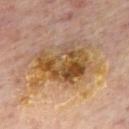Q: Was a biopsy performed?
A: imaged on a skin check; not biopsied
Q: Illumination type?
A: cross-polarized
Q: Patient demographics?
A: male, aged 63–67
Q: What is the lesion's diameter?
A: ≈5.5 mm
Q: Lesion location?
A: the mid back
Q: What kind of image is this?
A: total-body-photography crop, ~15 mm field of view